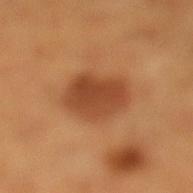Impression: Part of a total-body skin-imaging series; this lesion was reviewed on a skin check and was not flagged for biopsy. Clinical summary: About 5 mm across. A roughly 15 mm field-of-view crop from a total-body skin photograph. The lesion is on the left lower leg. A male patient, in their mid- to late 50s. Imaged with cross-polarized lighting.